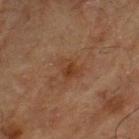biopsy status: no biopsy performed (imaged during a skin exam) | lesion diameter: about 2.5 mm | tile lighting: cross-polarized illumination | subject: male, in their mid- to late 60s | imaging modality: ~15 mm crop, total-body skin-cancer survey | location: the left lower leg.